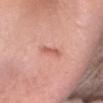| feature | finding |
|---|---|
| follow-up | imaged on a skin check; not biopsied |
| body site | the head or neck |
| imaging modality | total-body-photography crop, ~15 mm field of view |
| patient | female, aged 38 to 42 |